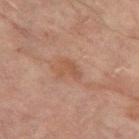A female subject, aged 78 to 82.
A 15 mm close-up extracted from a 3D total-body photography capture.
The lesion is on the leg.